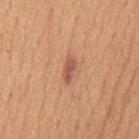biopsy status = total-body-photography surveillance lesion; no biopsy | imaging modality = total-body-photography crop, ~15 mm field of view | location = the back | patient = male, about 55 years old.